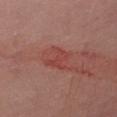{
  "biopsy_status": "not biopsied; imaged during a skin examination",
  "patient": {
    "sex": "female",
    "age_approx": 30
  },
  "lighting": "white-light",
  "site": "right thigh",
  "image": {
    "source": "total-body photography crop",
    "field_of_view_mm": 15
  }
}